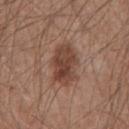Impression: This lesion was catalogued during total-body skin photography and was not selected for biopsy. Background: Automated tile analysis of the lesion measured a lesion color around L≈43 a*≈20 b*≈26 in CIELAB and about 11 CIELAB-L* units darker than the surrounding skin. And it measured a within-lesion color-variation index near 5/10 and a peripheral color-asymmetry measure near 1.5. Measured at roughly 5.5 mm in maximum diameter. The lesion is on the abdomen. The subject is a male in their mid-60s. Imaged with white-light lighting. A close-up tile cropped from a whole-body skin photograph, about 15 mm across.